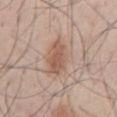workup: imaged on a skin check; not biopsied | automated lesion analysis: about 8 CIELAB-L* units darker than the surrounding skin and a normalized border contrast of about 5.5; a border-irregularity index near 3.5/10 and radial color variation of about 2 | imaging modality: 15 mm crop, total-body photography | patient: male, roughly 30 years of age | body site: the front of the torso | diameter: ~6.5 mm (longest diameter).